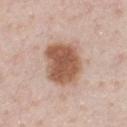Findings:
– notes: imaged on a skin check; not biopsied
– lesion size: ~5.5 mm (longest diameter)
– image: total-body-photography crop, ~15 mm field of view
– tile lighting: white-light illumination
– anatomic site: the chest
– patient: male, aged 58–62